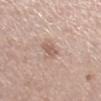No biopsy was performed on this lesion — it was imaged during a full skin examination and was not determined to be concerning. The lesion is located on the right lower leg. A roughly 15 mm field-of-view crop from a total-body skin photograph. A female patient, roughly 60 years of age.